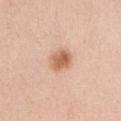Impression: The lesion was photographed on a routine skin check and not biopsied; there is no pathology result. Clinical summary: A 15 mm crop from a total-body photograph taken for skin-cancer surveillance. Located on the abdomen. A female patient, aged 48–52. Automated tile analysis of the lesion measured a shape eccentricity near 0.55 and a symmetry-axis asymmetry near 0.2. The analysis additionally found a lesion-detection confidence of about 100/100. The lesion's longest dimension is about 3 mm.